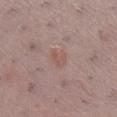Impression:
The lesion was tiled from a total-body skin photograph and was not biopsied.
Background:
Automated image analysis of the tile measured a border-irregularity index near 2/10 and a within-lesion color-variation index near 2/10. A 15 mm close-up extracted from a 3D total-body photography capture. The recorded lesion diameter is about 3 mm. This is a white-light tile. The lesion is located on the right lower leg. A female patient in their mid- to late 50s.